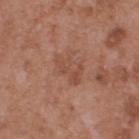Findings:
* biopsy status · catalogued during a skin exam; not biopsied
* site · the upper back
* size · ~4 mm (longest diameter)
* patient · male, in their mid- to late 50s
* image-analysis metrics · an area of roughly 6.5 mm², an eccentricity of roughly 0.8, and a symmetry-axis asymmetry near 0.35; an automated nevus-likeness rating near 0 out of 100
* acquisition · total-body-photography crop, ~15 mm field of view